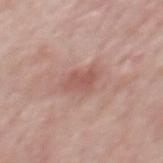Part of a total-body skin-imaging series; this lesion was reviewed on a skin check and was not flagged for biopsy. A lesion tile, about 15 mm wide, cut from a 3D total-body photograph. Approximately 3.5 mm at its widest. The lesion is located on the mid back. A male subject aged 53–57. The lesion-visualizer software estimated an area of roughly 6 mm² and a shape eccentricity near 0.7. The analysis additionally found a detector confidence of about 100 out of 100 that the crop contains a lesion. Imaged with white-light lighting.